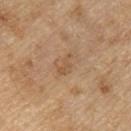Part of a total-body skin-imaging series; this lesion was reviewed on a skin check and was not flagged for biopsy.
The lesion-visualizer software estimated an outline eccentricity of about 0.9 (0 = round, 1 = elongated) and a shape-asymmetry score of about 0.4 (0 = symmetric). It also reported border irregularity of about 4.5 on a 0–10 scale, a color-variation rating of about 0.5/10, and peripheral color asymmetry of about 0.
From the front of the torso.
Longest diameter approximately 3 mm.
The patient is a male about 70 years old.
The tile uses white-light illumination.
A 15 mm crop from a total-body photograph taken for skin-cancer surveillance.